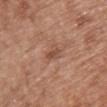The lesion was photographed on a routine skin check and not biopsied; there is no pathology result. Automated image analysis of the tile measured a lesion area of about 3.5 mm² and a shape-asymmetry score of about 0.45 (0 = symmetric). It also reported an average lesion color of about L≈50 a*≈22 b*≈29 (CIELAB), a lesion–skin lightness drop of about 8, and a normalized lesion–skin contrast near 6. The software also gave a classifier nevus-likeness of about 0/100. Approximately 3 mm at its widest. Cropped from a whole-body photographic skin survey; the tile spans about 15 mm. Located on the front of the torso. The subject is a female aged 38 to 42. This is a white-light tile.